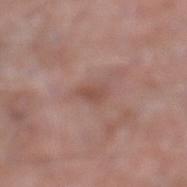Impression: Part of a total-body skin-imaging series; this lesion was reviewed on a skin check and was not flagged for biopsy. Clinical summary: Automated tile analysis of the lesion measured an area of roughly 3 mm² and a shape-asymmetry score of about 0.3 (0 = symmetric). And it measured a border-irregularity rating of about 3/10, a color-variation rating of about 1/10, and a peripheral color-asymmetry measure near 0.5. A male patient, aged around 75. The lesion is on the left lower leg. Captured under white-light illumination. The recorded lesion diameter is about 2.5 mm. Cropped from a total-body skin-imaging series; the visible field is about 15 mm.